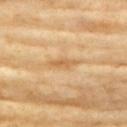{"biopsy_status": "not biopsied; imaged during a skin examination", "site": "chest", "patient": {"sex": "female", "age_approx": 75}, "image": {"source": "total-body photography crop", "field_of_view_mm": 15}, "automated_metrics": {"area_mm2_approx": 3.5, "eccentricity": 0.95, "cielab_L": 64, "cielab_a": 19, "cielab_b": 42, "vs_skin_darker_L": 8.0, "vs_skin_contrast_norm": 6.0, "nevus_likeness_0_100": 0}, "lighting": "cross-polarized"}